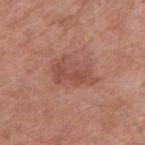notes=no biopsy performed (imaged during a skin exam); image=~15 mm tile from a whole-body skin photo; subject=male, aged approximately 55; illumination=white-light illumination; lesion diameter=about 5 mm; location=the right upper arm.